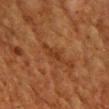Captured during whole-body skin photography for melanoma surveillance; the lesion was not biopsied.
On the chest.
A male patient, aged around 60.
Automated tile analysis of the lesion measured a lesion color around L≈31 a*≈21 b*≈31 in CIELAB, a lesion–skin lightness drop of about 6, and a normalized border contrast of about 6. And it measured an automated nevus-likeness rating near 0 out of 100 and lesion-presence confidence of about 100/100.
Longest diameter approximately 3 mm.
Imaged with cross-polarized lighting.
Cropped from a whole-body photographic skin survey; the tile spans about 15 mm.